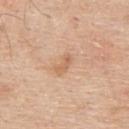Q: Was this lesion biopsied?
A: catalogued during a skin exam; not biopsied
Q: What is the anatomic site?
A: the upper back
Q: Who is the patient?
A: male, aged 58 to 62
Q: Lesion size?
A: about 2.5 mm
Q: How was the tile lit?
A: white-light
Q: What is the imaging modality?
A: ~15 mm crop, total-body skin-cancer survey
Q: Automated lesion metrics?
A: border irregularity of about 4.5 on a 0–10 scale, a color-variation rating of about 0/10, and a peripheral color-asymmetry measure near 0; a nevus-likeness score of about 15/100 and a lesion-detection confidence of about 100/100